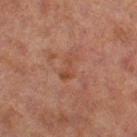workup: imaged on a skin check; not biopsied | subject: female, roughly 60 years of age | size: about 3.5 mm | tile lighting: cross-polarized | imaging modality: ~15 mm tile from a whole-body skin photo | anatomic site: the right thigh.